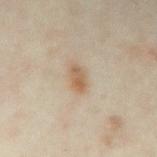Clinical impression: No biopsy was performed on this lesion — it was imaged during a full skin examination and was not determined to be concerning. Image and clinical context: This is a cross-polarized tile. Automated tile analysis of the lesion measured a lesion area of about 4.5 mm² and a shape eccentricity near 0.85. The software also gave an average lesion color of about L≈57 a*≈15 b*≈31 (CIELAB) and about 9 CIELAB-L* units darker than the surrounding skin. Approximately 3 mm at its widest. The lesion is located on the arm. Cropped from a total-body skin-imaging series; the visible field is about 15 mm. A female subject about 35 years old.